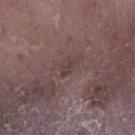No biopsy was performed on this lesion — it was imaged during a full skin examination and was not determined to be concerning.
The tile uses white-light illumination.
A male subject, aged around 75.
A roughly 15 mm field-of-view crop from a total-body skin photograph.
Located on the right lower leg.